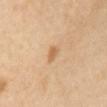The lesion was photographed on a routine skin check and not biopsied; there is no pathology result.
Captured under cross-polarized illumination.
The total-body-photography lesion software estimated a nevus-likeness score of about 65/100 and lesion-presence confidence of about 100/100.
A female patient, aged 53 to 57.
A roughly 15 mm field-of-view crop from a total-body skin photograph.
The lesion is on the abdomen.
The lesion's longest dimension is about 3 mm.